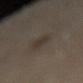{"biopsy_status": "not biopsied; imaged during a skin examination", "site": "lower back", "image": {"source": "total-body photography crop", "field_of_view_mm": 15}, "lighting": "cross-polarized", "patient": {"sex": "female", "age_approx": 65}, "lesion_size": {"long_diameter_mm_approx": 3.5}, "automated_metrics": {"cielab_L": 32, "cielab_a": 8, "cielab_b": 18, "vs_skin_contrast_norm": 6.0, "nevus_likeness_0_100": 0, "lesion_detection_confidence_0_100": 95}}